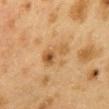follow-up: no biopsy performed (imaged during a skin exam) | location: the back | illumination: cross-polarized | imaging modality: ~15 mm crop, total-body skin-cancer survey | size: ~4 mm (longest diameter) | patient: female, aged around 40.